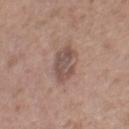  biopsy_status: not biopsied; imaged during a skin examination
  image:
    source: total-body photography crop
    field_of_view_mm: 15
  site: right thigh
  patient:
    sex: female
    age_approx: 75
  lighting: white-light
  automated_metrics:
    lesion_detection_confidence_0_100: 100
  lesion_size:
    long_diameter_mm_approx: 4.0